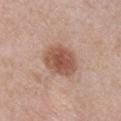Assessment: Recorded during total-body skin imaging; not selected for excision or biopsy. Context: The lesion is located on the chest. A lesion tile, about 15 mm wide, cut from a 3D total-body photograph. The lesion-visualizer software estimated a mean CIELAB color near L≈53 a*≈21 b*≈29 and a normalized lesion–skin contrast near 9. The software also gave a classifier nevus-likeness of about 95/100. The patient is a female aged 48 to 52. The recorded lesion diameter is about 4.5 mm. The tile uses white-light illumination.